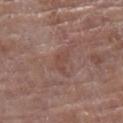biopsy_status: not biopsied; imaged during a skin examination
patient:
  sex: female
  age_approx: 80
lighting: white-light
site: left lower leg
lesion_size:
  long_diameter_mm_approx: 3.0
image:
  source: total-body photography crop
  field_of_view_mm: 15